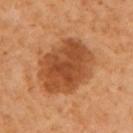Clinical impression: The lesion was photographed on a routine skin check and not biopsied; there is no pathology result. Background: Approximately 7.5 mm at its widest. Cropped from a total-body skin-imaging series; the visible field is about 15 mm. On the left upper arm. A female patient in their mid- to late 50s. Captured under cross-polarized illumination.